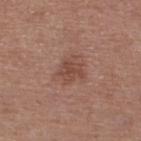  biopsy_status: not biopsied; imaged during a skin examination
  image:
    source: total-body photography crop
    field_of_view_mm: 15
  site: left lower leg
  lighting: white-light
  patient:
    sex: female
    age_approx: 55
  automated_metrics:
    vs_skin_darker_L: 8.0
  lesion_size:
    long_diameter_mm_approx: 3.0